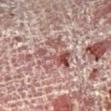lesion size = about 5 mm; patient = male, in their mid- to late 50s; tile lighting = cross-polarized; anatomic site = the right lower leg; image = 15 mm crop, total-body photography.